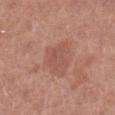Impression: The lesion was tiled from a total-body skin photograph and was not biopsied. Image and clinical context: A female patient approximately 50 years of age. Captured under white-light illumination. This image is a 15 mm lesion crop taken from a total-body photograph. Automated tile analysis of the lesion measured a lesion area of about 6 mm², an eccentricity of roughly 0.65, and two-axis asymmetry of about 0.35. And it measured a mean CIELAB color near L≈52 a*≈24 b*≈25, roughly 6 lightness units darker than nearby skin, and a normalized lesion–skin contrast near 4.5. The analysis additionally found a nevus-likeness score of about 0/100 and a lesion-detection confidence of about 100/100. From the right lower leg.